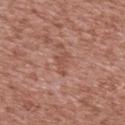Clinical impression: The lesion was tiled from a total-body skin photograph and was not biopsied. Context: Measured at roughly 3 mm in maximum diameter. The lesion is located on the upper back. A male patient, in their mid-40s. A 15 mm close-up extracted from a 3D total-body photography capture.